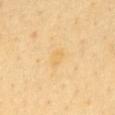Assessment: No biopsy was performed on this lesion — it was imaged during a full skin examination and was not determined to be concerning. Clinical summary: This is a cross-polarized tile. The lesion-visualizer software estimated an average lesion color of about L≈75 a*≈15 b*≈48 (CIELAB), a lesion–skin lightness drop of about 5, and a lesion-to-skin contrast of about 4.5 (normalized; higher = more distinct). And it measured border irregularity of about 2 on a 0–10 scale, a within-lesion color-variation index near 2.5/10, and a peripheral color-asymmetry measure near 1. On the abdomen. Cropped from a whole-body photographic skin survey; the tile spans about 15 mm. A male subject aged approximately 65.